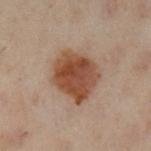Imaged during a routine full-body skin examination; the lesion was not biopsied and no histopathology is available. Cropped from a whole-body photographic skin survey; the tile spans about 15 mm. From the leg. The tile uses cross-polarized illumination. The recorded lesion diameter is about 5 mm. A female subject, aged 58–62. The total-body-photography lesion software estimated a mean CIELAB color near L≈40 a*≈19 b*≈27, about 12 CIELAB-L* units darker than the surrounding skin, and a normalized lesion–skin contrast near 10.5. It also reported internal color variation of about 5 on a 0–10 scale.